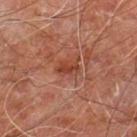Impression: Part of a total-body skin-imaging series; this lesion was reviewed on a skin check and was not flagged for biopsy. Background: Approximately 3 mm at its widest. Located on the right leg. A region of skin cropped from a whole-body photographic capture, roughly 15 mm wide. A male subject roughly 60 years of age.